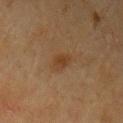workup — no biopsy performed (imaged during a skin exam)
illumination — cross-polarized
site — the left upper arm
patient — female, in their 80s
image-analysis metrics — a lesion area of about 4 mm² and an eccentricity of roughly 0.8; an average lesion color of about L≈34 a*≈17 b*≈29 (CIELAB), a lesion–skin lightness drop of about 6, and a lesion-to-skin contrast of about 6.5 (normalized; higher = more distinct); an automated nevus-likeness rating near 75 out of 100
lesion size — ≈2.5 mm
acquisition — ~15 mm crop, total-body skin-cancer survey